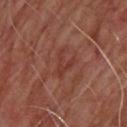Recorded during total-body skin imaging; not selected for excision or biopsy.
A lesion tile, about 15 mm wide, cut from a 3D total-body photograph.
The lesion is located on the back.
A male subject, in their mid- to late 60s.
The recorded lesion diameter is about 3.5 mm.